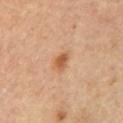Impression: This lesion was catalogued during total-body skin photography and was not selected for biopsy. Context: About 2.5 mm across. A lesion tile, about 15 mm wide, cut from a 3D total-body photograph. The lesion is on the left upper arm. The patient is a male in their mid- to late 50s. Imaged with cross-polarized lighting.